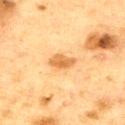Imaged during a routine full-body skin examination; the lesion was not biopsied and no histopathology is available. Cropped from a whole-body photographic skin survey; the tile spans about 15 mm. Located on the chest. Captured under cross-polarized illumination. Approximately 3.5 mm at its widest. The subject is a male approximately 65 years of age.